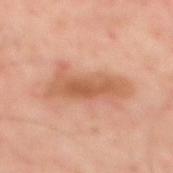Findings:
* notes · imaged on a skin check; not biopsied
* lesion diameter · ~7.5 mm (longest diameter)
* patient · male, about 50 years old
* illumination · cross-polarized
* anatomic site · the mid back
* imaging modality · ~15 mm tile from a whole-body skin photo